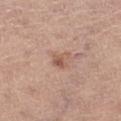Q: What kind of image is this?
A: total-body-photography crop, ~15 mm field of view
Q: Lesion location?
A: the left thigh
Q: Illumination type?
A: white-light illumination
Q: How large is the lesion?
A: ~2.5 mm (longest diameter)
Q: What are the patient's age and sex?
A: female, aged around 65
Q: Automated lesion metrics?
A: an average lesion color of about L≈56 a*≈20 b*≈29 (CIELAB), roughly 9 lightness units darker than nearby skin, and a normalized border contrast of about 6.5; an automated nevus-likeness rating near 25 out of 100 and lesion-presence confidence of about 100/100